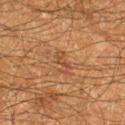Q: Was a biopsy performed?
A: catalogued during a skin exam; not biopsied
Q: What is the anatomic site?
A: the right lower leg
Q: What lighting was used for the tile?
A: cross-polarized
Q: What did automated image analysis measure?
A: a lesion area of about 3.5 mm² and two-axis asymmetry of about 0.4; roughly 6 lightness units darker than nearby skin and a normalized border contrast of about 5.5; a border-irregularity index near 5/10 and a color-variation rating of about 0/10
Q: What is the imaging modality?
A: ~15 mm crop, total-body skin-cancer survey
Q: Patient demographics?
A: male, about 60 years old